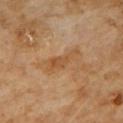Imaged during a routine full-body skin examination; the lesion was not biopsied and no histopathology is available.
From the front of the torso.
The total-body-photography lesion software estimated a classifier nevus-likeness of about 0/100 and a detector confidence of about 100 out of 100 that the crop contains a lesion.
A male patient roughly 60 years of age.
A close-up tile cropped from a whole-body skin photograph, about 15 mm across.
The lesion's longest dimension is about 4.5 mm.